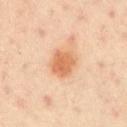workup: no biopsy performed (imaged during a skin exam)
imaging modality: ~15 mm crop, total-body skin-cancer survey
image-analysis metrics: an area of roughly 8 mm², a shape eccentricity near 0.45, and a shape-asymmetry score of about 0.2 (0 = symmetric); a border-irregularity rating of about 2/10, internal color variation of about 3.5 on a 0–10 scale, and peripheral color asymmetry of about 1.5; lesion-presence confidence of about 100/100
illumination: cross-polarized
patient: male, roughly 50 years of age
body site: the left upper arm
diameter: ≈3.5 mm